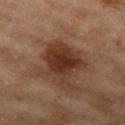This lesion was catalogued during total-body skin photography and was not selected for biopsy. Captured under cross-polarized illumination. About 5 mm across. Automated tile analysis of the lesion measured a lesion color around L≈30 a*≈19 b*≈26 in CIELAB, a lesion–skin lightness drop of about 10, and a normalized lesion–skin contrast near 10. And it measured a border-irregularity index near 4.5/10 and a within-lesion color-variation index near 3.5/10. It also reported an automated nevus-likeness rating near 90 out of 100 and lesion-presence confidence of about 100/100. From the left thigh. The subject is a female in their 60s. A region of skin cropped from a whole-body photographic capture, roughly 15 mm wide.